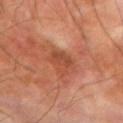Clinical impression:
No biopsy was performed on this lesion — it was imaged during a full skin examination and was not determined to be concerning.
Clinical summary:
A close-up tile cropped from a whole-body skin photograph, about 15 mm across. This is a cross-polarized tile. The lesion-visualizer software estimated a lesion area of about 7 mm², a shape eccentricity near 0.2, and a shape-asymmetry score of about 0.3 (0 = symmetric). Approximately 3 mm at its widest. The patient is a male aged around 70. Located on the arm.